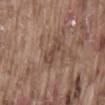| field | value |
|---|---|
| biopsy status | imaged on a skin check; not biopsied |
| automated metrics | an area of roughly 4 mm² and a symmetry-axis asymmetry near 0.4 |
| size | about 3.5 mm |
| anatomic site | the lower back |
| lighting | white-light |
| image source | 15 mm crop, total-body photography |
| subject | male, approximately 75 years of age |